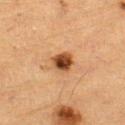Q: Was a biopsy performed?
A: imaged on a skin check; not biopsied
Q: What did automated image analysis measure?
A: a detector confidence of about 100 out of 100 that the crop contains a lesion
Q: What kind of image is this?
A: 15 mm crop, total-body photography
Q: How was the tile lit?
A: cross-polarized illumination
Q: What is the lesion's diameter?
A: about 2.5 mm
Q: Where on the body is the lesion?
A: the leg
Q: Patient demographics?
A: male, aged approximately 85 or older